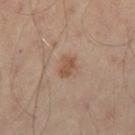| feature | finding |
|---|---|
| follow-up | catalogued during a skin exam; not biopsied |
| lighting | cross-polarized |
| lesion size | ~2.5 mm (longest diameter) |
| subject | male, in their 50s |
| acquisition | ~15 mm tile from a whole-body skin photo |
| automated lesion analysis | a lesion color around L≈50 a*≈18 b*≈29 in CIELAB, a lesion–skin lightness drop of about 8, and a normalized border contrast of about 7; internal color variation of about 1.5 on a 0–10 scale and peripheral color asymmetry of about 0.5; an automated nevus-likeness rating near 65 out of 100 and a detector confidence of about 100 out of 100 that the crop contains a lesion |
| site | the left leg |